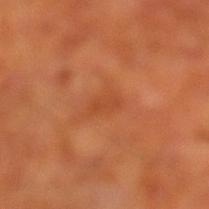The lesion was tiled from a total-body skin photograph and was not biopsied.
A lesion tile, about 15 mm wide, cut from a 3D total-body photograph.
The recorded lesion diameter is about 3 mm.
The lesion is on the left lower leg.
The subject is a male in their 70s.
This is a cross-polarized tile.
Automated tile analysis of the lesion measured a mean CIELAB color near L≈46 a*≈29 b*≈39 and a normalized border contrast of about 4.5. And it measured a border-irregularity rating of about 3.5/10, a within-lesion color-variation index near 1.5/10, and peripheral color asymmetry of about 0.5.